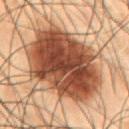biopsy_status: not biopsied; imaged during a skin examination